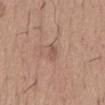Notes:
* follow-up — catalogued during a skin exam; not biopsied
* patient — male, roughly 65 years of age
* location — the mid back
* illumination — white-light
* image-analysis metrics — a lesion area of about 3 mm² and a shape-asymmetry score of about 0.3 (0 = symmetric); a border-irregularity rating of about 3.5/10 and a peripheral color-asymmetry measure near 0; a classifier nevus-likeness of about 0/100 and a detector confidence of about 100 out of 100 that the crop contains a lesion
* acquisition — ~15 mm crop, total-body skin-cancer survey